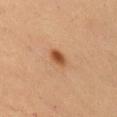A 15 mm close-up extracted from a 3D total-body photography capture.
The lesion is located on the right upper arm.
The patient is a male about 55 years old.
Captured under cross-polarized illumination.
The total-body-photography lesion software estimated a lesion–skin lightness drop of about 12. It also reported lesion-presence confidence of about 100/100.
The lesion's longest dimension is about 2.5 mm.